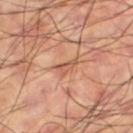Recorded during total-body skin imaging; not selected for excision or biopsy. Located on the left thigh. The lesion's longest dimension is about 2.5 mm. A male patient about 60 years old. A lesion tile, about 15 mm wide, cut from a 3D total-body photograph.